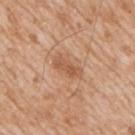Captured during whole-body skin photography for melanoma surveillance; the lesion was not biopsied. Captured under white-light illumination. The total-body-photography lesion software estimated a footprint of about 5 mm², an outline eccentricity of about 0.9 (0 = round, 1 = elongated), and a symmetry-axis asymmetry near 0.35. The software also gave a border-irregularity index near 3.5/10 and a within-lesion color-variation index near 2.5/10. A male subject about 65 years old. Longest diameter approximately 3.5 mm. Cropped from a whole-body photographic skin survey; the tile spans about 15 mm. On the right upper arm.